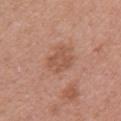Imaged during a routine full-body skin examination; the lesion was not biopsied and no histopathology is available. Imaged with white-light lighting. A close-up tile cropped from a whole-body skin photograph, about 15 mm across. Longest diameter approximately 3 mm. A female patient, roughly 40 years of age. The total-body-photography lesion software estimated a lesion area of about 6 mm² and an eccentricity of roughly 0.4. The software also gave a lesion color around L≈53 a*≈23 b*≈30 in CIELAB, about 8 CIELAB-L* units darker than the surrounding skin, and a normalized lesion–skin contrast near 5.5. And it measured a border-irregularity rating of about 3.5/10, a color-variation rating of about 2.5/10, and radial color variation of about 1. The lesion is located on the right upper arm.